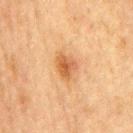Part of a total-body skin-imaging series; this lesion was reviewed on a skin check and was not flagged for biopsy. The total-body-photography lesion software estimated a lesion area of about 6.5 mm², an outline eccentricity of about 0.65 (0 = round, 1 = elongated), and a shape-asymmetry score of about 0.25 (0 = symmetric). And it measured a border-irregularity index near 2.5/10, a within-lesion color-variation index near 5/10, and radial color variation of about 2. It also reported an automated nevus-likeness rating near 90 out of 100. Measured at roughly 3.5 mm in maximum diameter. The patient is a male aged 73–77. A lesion tile, about 15 mm wide, cut from a 3D total-body photograph. Located on the chest.